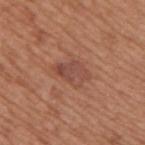{
  "biopsy_status": "not biopsied; imaged during a skin examination",
  "automated_metrics": {
    "border_irregularity_0_10": 3.0,
    "color_variation_0_10": 4.0,
    "nevus_likeness_0_100": 30,
    "lesion_detection_confidence_0_100": 100
  },
  "lighting": "white-light",
  "patient": {
    "sex": "male",
    "age_approx": 65
  },
  "lesion_size": {
    "long_diameter_mm_approx": 4.0
  },
  "image": {
    "source": "total-body photography crop",
    "field_of_view_mm": 15
  },
  "site": "back"
}